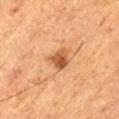{
  "biopsy_status": "not biopsied; imaged during a skin examination",
  "patient": {
    "sex": "male",
    "age_approx": 75
  },
  "lesion_size": {
    "long_diameter_mm_approx": 3.0
  },
  "automated_metrics": {
    "vs_skin_darker_L": 11.0,
    "vs_skin_contrast_norm": 9.0,
    "border_irregularity_0_10": 2.5,
    "color_variation_0_10": 5.0
  },
  "image": {
    "source": "total-body photography crop",
    "field_of_view_mm": 15
  },
  "site": "leg"
}